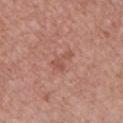{"biopsy_status": "not biopsied; imaged during a skin examination", "patient": {"sex": "female", "age_approx": 45}, "site": "chest", "lesion_size": {"long_diameter_mm_approx": 3.0}, "image": {"source": "total-body photography crop", "field_of_view_mm": 15}}